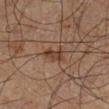biopsy status: total-body-photography surveillance lesion; no biopsy | imaging modality: 15 mm crop, total-body photography | subject: male, roughly 65 years of age | site: the right lower leg | automated lesion analysis: an area of roughly 3.5 mm² and an eccentricity of roughly 0.85; an average lesion color of about L≈31 a*≈15 b*≈23 (CIELAB), about 8 CIELAB-L* units darker than the surrounding skin, and a normalized lesion–skin contrast near 8.5; a border-irregularity index near 4/10, a within-lesion color-variation index near 0.5/10, and radial color variation of about 0; a classifier nevus-likeness of about 25/100 and a detector confidence of about 100 out of 100 that the crop contains a lesion | tile lighting: cross-polarized | size: about 3 mm.